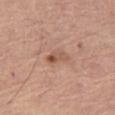<case>
  <biopsy_status>not biopsied; imaged during a skin examination</biopsy_status>
  <patient>
    <sex>male</sex>
    <age_approx>65</age_approx>
  </patient>
  <site>left thigh</site>
  <lesion_size>
    <long_diameter_mm_approx>3.5</long_diameter_mm_approx>
  </lesion_size>
  <image>
    <source>total-body photography crop</source>
    <field_of_view_mm>15</field_of_view_mm>
  </image>
  <lighting>white-light</lighting>
</case>